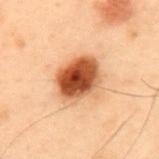  biopsy_status: not biopsied; imaged during a skin examination
  patient:
    sex: male
    age_approx: 55
  site: back
  automated_metrics:
    area_mm2_approx: 17.0
    eccentricity: 0.6
    shape_asymmetry: 0.2
  lesion_size:
    long_diameter_mm_approx: 5.0
  lighting: cross-polarized
  image:
    source: total-body photography crop
    field_of_view_mm: 15